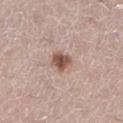• workup · catalogued during a skin exam; not biopsied
• tile lighting · white-light illumination
• acquisition · total-body-photography crop, ~15 mm field of view
• location · the leg
• diameter · ≈3 mm
• patient · female, about 40 years old